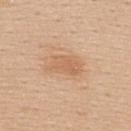Imaged during a routine full-body skin examination; the lesion was not biopsied and no histopathology is available. From the back. A 15 mm close-up extracted from a 3D total-body photography capture. The recorded lesion diameter is about 4.5 mm. The patient is a female aged 43–47. Automated tile analysis of the lesion measured a nevus-likeness score of about 60/100 and a lesion-detection confidence of about 100/100.